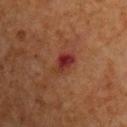biopsy status: no biopsy performed (imaged during a skin exam) | lighting: cross-polarized illumination | image source: ~15 mm tile from a whole-body skin photo | diameter: ~3.5 mm (longest diameter) | automated metrics: an area of roughly 5.5 mm², an outline eccentricity of about 0.85 (0 = round, 1 = elongated), and a symmetry-axis asymmetry near 0.25; a mean CIELAB color near L≈29 a*≈25 b*≈25, roughly 8 lightness units darker than nearby skin, and a normalized border contrast of about 8.5 | patient: male, in their mid- to late 60s | location: the front of the torso.